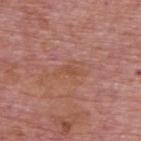Part of a total-body skin-imaging series; this lesion was reviewed on a skin check and was not flagged for biopsy.
The tile uses white-light illumination.
A close-up tile cropped from a whole-body skin photograph, about 15 mm across.
On the back.
An algorithmic analysis of the crop reported an area of roughly 2.5 mm², a shape eccentricity near 0.9, and a symmetry-axis asymmetry near 0.55. The analysis additionally found an automated nevus-likeness rating near 0 out of 100 and a lesion-detection confidence of about 100/100.
The subject is a male aged approximately 75.